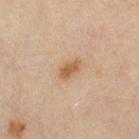Impression: Part of a total-body skin-imaging series; this lesion was reviewed on a skin check and was not flagged for biopsy. Clinical summary: The patient is a female in their 50s. A 15 mm close-up extracted from a 3D total-body photography capture. The lesion is located on the right thigh. About 3 mm across.